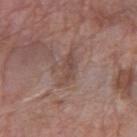Image and clinical context:
The recorded lesion diameter is about 3.5 mm. A 15 mm close-up extracted from a 3D total-body photography capture. A female patient aged 68–72. The total-body-photography lesion software estimated an area of roughly 4.5 mm², a shape eccentricity near 0.9, and two-axis asymmetry of about 0.35. It also reported a mean CIELAB color near L≈46 a*≈18 b*≈23 and a normalized lesion–skin contrast near 6. And it measured a classifier nevus-likeness of about 0/100 and a lesion-detection confidence of about 100/100. From the right forearm. Imaged with white-light lighting.